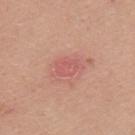Q: Who is the patient?
A: male, aged around 25
Q: Where on the body is the lesion?
A: the upper back
Q: What kind of image is this?
A: ~15 mm tile from a whole-body skin photo